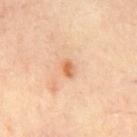  biopsy_status: not biopsied; imaged during a skin examination
  patient:
    sex: male
    age_approx: 65
  image:
    source: total-body photography crop
    field_of_view_mm: 15
  site: chest
  automated_metrics:
    area_mm2_approx: 2.5
    eccentricity: 0.7
    shape_asymmetry: 0.25
    nevus_likeness_0_100: 60
    lesion_detection_confidence_0_100: 100